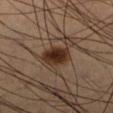<record>
  <biopsy_status>not biopsied; imaged during a skin examination</biopsy_status>
  <site>right lower leg</site>
  <patient>
    <sex>male</sex>
    <age_approx>65</age_approx>
  </patient>
  <image>
    <source>total-body photography crop</source>
    <field_of_view_mm>15</field_of_view_mm>
  </image>
</record>